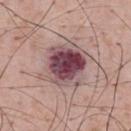{"biopsy_status": "not biopsied; imaged during a skin examination", "image": {"source": "total-body photography crop", "field_of_view_mm": 15}, "patient": {"sex": "male", "age_approx": 55}, "lesion_size": {"long_diameter_mm_approx": 5.5}, "lighting": "white-light", "automated_metrics": {"nevus_likeness_0_100": 15}, "site": "upper back"}